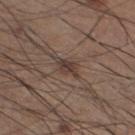Assessment:
The lesion was tiled from a total-body skin photograph and was not biopsied.
Acquisition and patient details:
About 3 mm across. The lesion is located on the left lower leg. Imaged with white-light lighting. A male patient in their mid-30s. The total-body-photography lesion software estimated a shape eccentricity near 0.8 and a symmetry-axis asymmetry near 0.35. The analysis additionally found a detector confidence of about 95 out of 100 that the crop contains a lesion. A close-up tile cropped from a whole-body skin photograph, about 15 mm across.